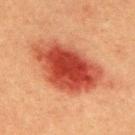Imaged during a routine full-body skin examination; the lesion was not biopsied and no histopathology is available. Automated tile analysis of the lesion measured a lesion area of about 33 mm² and a shape-asymmetry score of about 0.2 (0 = symmetric). It also reported a mean CIELAB color near L≈41 a*≈31 b*≈32, roughly 15 lightness units darker than nearby skin, and a normalized border contrast of about 11. The software also gave a nevus-likeness score of about 100/100. The lesion is on the back. This is a cross-polarized tile. A roughly 15 mm field-of-view crop from a total-body skin photograph. A male patient in their 40s.